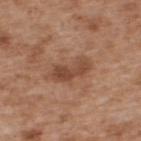Background:
The lesion-visualizer software estimated an area of roughly 8.5 mm², an eccentricity of roughly 0.85, and two-axis asymmetry of about 0.3. The software also gave a lesion color around L≈47 a*≈22 b*≈30 in CIELAB and about 9 CIELAB-L* units darker than the surrounding skin. It also reported a border-irregularity rating of about 3.5/10, a color-variation rating of about 3.5/10, and radial color variation of about 1.5. The software also gave a classifier nevus-likeness of about 15/100 and lesion-presence confidence of about 100/100. Longest diameter approximately 4.5 mm. A male patient, approximately 65 years of age. From the back. This image is a 15 mm lesion crop taken from a total-body photograph.